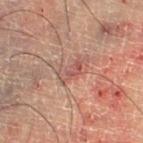Longest diameter approximately 3.5 mm. Captured under cross-polarized illumination. The total-body-photography lesion software estimated a mean CIELAB color near L≈46 a*≈22 b*≈23 and a normalized lesion–skin contrast near 6. A male subject, approximately 60 years of age. A 15 mm close-up tile from a total-body photography series done for melanoma screening.